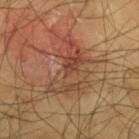Assessment:
Recorded during total-body skin imaging; not selected for excision or biopsy.
Image and clinical context:
Longest diameter approximately 6.5 mm. A male subject about 65 years old. Cropped from a total-body skin-imaging series; the visible field is about 15 mm. The lesion is on the left forearm. Captured under cross-polarized illumination.